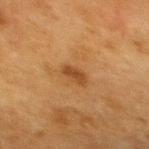| field | value |
|---|---|
| notes | imaged on a skin check; not biopsied |
| anatomic site | the upper back |
| lesion diameter | about 2.5 mm |
| patient | female, in their mid- to late 50s |
| automated metrics | a footprint of about 3.5 mm², an eccentricity of roughly 0.85, and a shape-asymmetry score of about 0.25 (0 = symmetric); an average lesion color of about L≈38 a*≈20 b*≈34 (CIELAB), about 8 CIELAB-L* units darker than the surrounding skin, and a normalized border contrast of about 7.5 |
| acquisition | 15 mm crop, total-body photography |